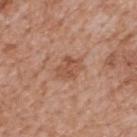This lesion was catalogued during total-body skin photography and was not selected for biopsy.
The lesion is located on the mid back.
Imaged with white-light lighting.
About 3 mm across.
A lesion tile, about 15 mm wide, cut from a 3D total-body photograph.
A male patient about 65 years old.